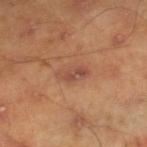Context: The lesion is on the leg. The patient is a male in their mid-40s. Measured at roughly 3.5 mm in maximum diameter. Captured under cross-polarized illumination. A lesion tile, about 15 mm wide, cut from a 3D total-body photograph.